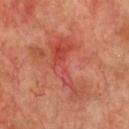Clinical impression: No biopsy was performed on this lesion — it was imaged during a full skin examination and was not determined to be concerning. Image and clinical context: A 15 mm crop from a total-body photograph taken for skin-cancer surveillance. Located on the chest. About 8 mm across. Imaged with cross-polarized lighting. Automated image analysis of the tile measured border irregularity of about 9 on a 0–10 scale and peripheral color asymmetry of about 1.5. A male patient aged 68–72.